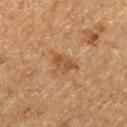Clinical impression: Part of a total-body skin-imaging series; this lesion was reviewed on a skin check and was not flagged for biopsy. Clinical summary: Automated tile analysis of the lesion measured an area of roughly 4.5 mm² and two-axis asymmetry of about 0.35. And it measured a mean CIELAB color near L≈40 a*≈17 b*≈30, a lesion–skin lightness drop of about 7, and a lesion-to-skin contrast of about 6.5 (normalized; higher = more distinct). The software also gave a border-irregularity rating of about 3.5/10, a color-variation rating of about 2/10, and radial color variation of about 0.5. And it measured an automated nevus-likeness rating near 0 out of 100 and a detector confidence of about 100 out of 100 that the crop contains a lesion. Captured under cross-polarized illumination. The recorded lesion diameter is about 3.5 mm. A 15 mm crop from a total-body photograph taken for skin-cancer surveillance. The lesion is located on the left thigh. A male subject aged approximately 75.